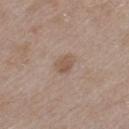biopsy status: imaged on a skin check; not biopsied
image: 15 mm crop, total-body photography
TBP lesion metrics: an area of roughly 4 mm², a shape eccentricity near 0.6, and a shape-asymmetry score of about 0.25 (0 = symmetric); a lesion-detection confidence of about 100/100
body site: the left thigh
lighting: white-light
subject: female, aged 38 to 42
size: ~2.5 mm (longest diameter)